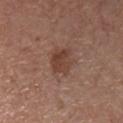Captured during whole-body skin photography for melanoma surveillance; the lesion was not biopsied. The subject is a male aged 53 to 57. The lesion is located on the chest. This is a white-light tile. A close-up tile cropped from a whole-body skin photograph, about 15 mm across.